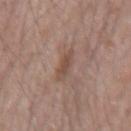A 15 mm close-up tile from a total-body photography series done for melanoma screening.
A male patient aged 48 to 52.
Located on the right forearm.
Longest diameter approximately 3.5 mm.
This is a white-light tile.
Automated image analysis of the tile measured a mean CIELAB color near L≈49 a*≈18 b*≈25 and a normalized lesion–skin contrast near 6.5. And it measured a border-irregularity rating of about 2/10, a within-lesion color-variation index near 1.5/10, and radial color variation of about 0.5.